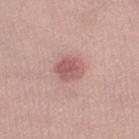follow-up = imaged on a skin check; not biopsied
imaging modality = 15 mm crop, total-body photography
tile lighting = white-light
patient = female, in their mid-20s
lesion diameter = ≈3.5 mm
anatomic site = the right lower leg